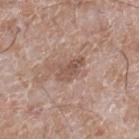follow-up: total-body-photography surveillance lesion; no biopsy | illumination: white-light | size: ≈4 mm | image: 15 mm crop, total-body photography | location: the right lower leg | subject: male, aged approximately 60 | TBP lesion metrics: internal color variation of about 2.5 on a 0–10 scale and radial color variation of about 1; a nevus-likeness score of about 0/100 and a detector confidence of about 100 out of 100 that the crop contains a lesion.